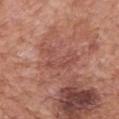- follow-up · imaged on a skin check; not biopsied
- patient · male, aged around 70
- illumination · white-light
- image · 15 mm crop, total-body photography
- TBP lesion metrics · an outline eccentricity of about 0.85 (0 = round, 1 = elongated) and a symmetry-axis asymmetry near 0.6
- site · the back